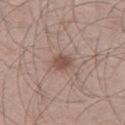Imaged during a routine full-body skin examination; the lesion was not biopsied and no histopathology is available.